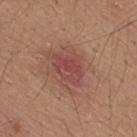Part of a total-body skin-imaging series; this lesion was reviewed on a skin check and was not flagged for biopsy.